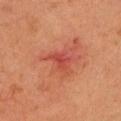The lesion was tiled from a total-body skin photograph and was not biopsied. Imaged with cross-polarized lighting. From the head or neck. A female patient aged 43–47. A 15 mm crop from a total-body photograph taken for skin-cancer surveillance. Automated tile analysis of the lesion measured a lesion area of about 4 mm², an outline eccentricity of about 0.65 (0 = round, 1 = elongated), and a symmetry-axis asymmetry near 0.45. The analysis additionally found a border-irregularity index near 4/10, a color-variation rating of about 2/10, and radial color variation of about 0.5. The software also gave a classifier nevus-likeness of about 0/100 and a lesion-detection confidence of about 85/100. The lesion's longest dimension is about 2.5 mm.